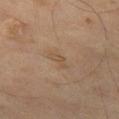<record>
  <biopsy_status>not biopsied; imaged during a skin examination</biopsy_status>
  <image>
    <source>total-body photography crop</source>
    <field_of_view_mm>15</field_of_view_mm>
  </image>
  <patient>
    <sex>male</sex>
    <age_approx>65</age_approx>
  </patient>
  <site>left thigh</site>
  <automated_metrics>
    <area_mm2_approx>3.0</area_mm2_approx>
    <eccentricity>0.9</eccentricity>
    <shape_asymmetry>0.35</shape_asymmetry>
    <border_irregularity_0_10>4.0</border_irregularity_0_10>
    <color_variation_0_10>0.5</color_variation_0_10>
    <peripheral_color_asymmetry>0.0</peripheral_color_asymmetry>
    <nevus_likeness_0_100>0</nevus_likeness_0_100>
    <lesion_detection_confidence_0_100>100</lesion_detection_confidence_0_100>
  </automated_metrics>
</record>